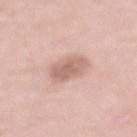<case>
  <biopsy_status>not biopsied; imaged during a skin examination</biopsy_status>
  <site>mid back</site>
  <automated_metrics>
    <nevus_likeness_0_100>10</nevus_likeness_0_100>
    <lesion_detection_confidence_0_100>100</lesion_detection_confidence_0_100>
  </automated_metrics>
  <patient>
    <sex>female</sex>
    <age_approx>70</age_approx>
  </patient>
  <image>
    <source>total-body photography crop</source>
    <field_of_view_mm>15</field_of_view_mm>
  </image>
</case>